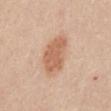Recorded during total-body skin imaging; not selected for excision or biopsy.
A female subject aged around 40.
Captured under white-light illumination.
A region of skin cropped from a whole-body photographic capture, roughly 15 mm wide.
The lesion is located on the mid back.
Measured at roughly 5 mm in maximum diameter.